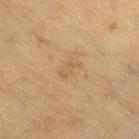Impression: Recorded during total-body skin imaging; not selected for excision or biopsy. Background: A lesion tile, about 15 mm wide, cut from a 3D total-body photograph. Longest diameter approximately 2.5 mm. The patient is a female roughly 55 years of age. The lesion is on the leg.